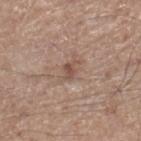Acquisition and patient details: A male patient roughly 65 years of age. The lesion is on the right lower leg. A 15 mm crop from a total-body photograph taken for skin-cancer surveillance. Imaged with white-light lighting. The lesion's longest dimension is about 2.5 mm.